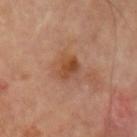This lesion was catalogued during total-body skin photography and was not selected for biopsy. The subject is a male aged 68–72. The recorded lesion diameter is about 3 mm. From the left upper arm. A lesion tile, about 15 mm wide, cut from a 3D total-body photograph.